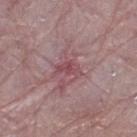<lesion>
<biopsy_status>not biopsied; imaged during a skin examination</biopsy_status>
<image>
  <source>total-body photography crop</source>
  <field_of_view_mm>15</field_of_view_mm>
</image>
<automated_metrics>
  <area_mm2_approx>3.5</area_mm2_approx>
  <eccentricity>0.85</eccentricity>
  <shape_asymmetry>0.35</shape_asymmetry>
  <cielab_L>47</cielab_L>
  <cielab_a>26</cielab_a>
  <cielab_b>16</cielab_b>
  <border_irregularity_0_10>3.5</border_irregularity_0_10>
  <color_variation_0_10>2.0</color_variation_0_10>
  <peripheral_color_asymmetry>0.5</peripheral_color_asymmetry>
  <nevus_likeness_0_100>0</nevus_likeness_0_100>
  <lesion_detection_confidence_0_100>75</lesion_detection_confidence_0_100>
</automated_metrics>
<lesion_size>
  <long_diameter_mm_approx>3.0</long_diameter_mm_approx>
</lesion_size>
<patient>
  <sex>female</sex>
  <age_approx>65</age_approx>
</patient>
<lighting>white-light</lighting>
<site>right lower leg</site>
</lesion>